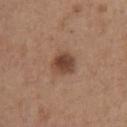Impression:
The lesion was photographed on a routine skin check and not biopsied; there is no pathology result.
Image and clinical context:
Automated tile analysis of the lesion measured an eccentricity of roughly 0.25 and a symmetry-axis asymmetry near 0.2. A male patient approximately 65 years of age. A 15 mm close-up extracted from a 3D total-body photography capture. The lesion's longest dimension is about 2.5 mm. The lesion is located on the chest. The tile uses white-light illumination.